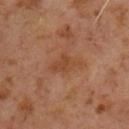No biopsy was performed on this lesion — it was imaged during a full skin examination and was not determined to be concerning. Approximately 3.5 mm at its widest. The lesion is on the chest. A male patient, aged 58 to 62. This image is a 15 mm lesion crop taken from a total-body photograph.